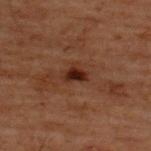Clinical summary:
A male patient, approximately 60 years of age. A 15 mm close-up tile from a total-body photography series done for melanoma screening. This is a cross-polarized tile. The lesion is located on the back.